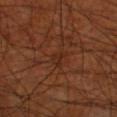{
  "biopsy_status": "not biopsied; imaged during a skin examination",
  "lesion_size": {
    "long_diameter_mm_approx": 2.5
  },
  "patient": {
    "sex": "male",
    "age_approx": 70
  },
  "image": {
    "source": "total-body photography crop",
    "field_of_view_mm": 15
  },
  "site": "right thigh",
  "lighting": "cross-polarized"
}